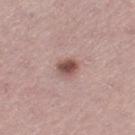<lesion>
  <biopsy_status>not biopsied; imaged during a skin examination</biopsy_status>
  <site>left thigh</site>
  <lighting>white-light</lighting>
  <image>
    <source>total-body photography crop</source>
    <field_of_view_mm>15</field_of_view_mm>
  </image>
  <patient>
    <sex>female</sex>
    <age_approx>40</age_approx>
  </patient>
  <lesion_size>
    <long_diameter_mm_approx>2.5</long_diameter_mm_approx>
  </lesion_size>
</lesion>